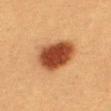Cropped from a whole-body photographic skin survey; the tile spans about 15 mm.
Located on the right thigh.
The tile uses cross-polarized illumination.
The patient is a female aged approximately 40.